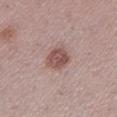The lesion was tiled from a total-body skin photograph and was not biopsied.
Imaged with white-light lighting.
The lesion's longest dimension is about 3.5 mm.
The total-body-photography lesion software estimated border irregularity of about 2 on a 0–10 scale, a within-lesion color-variation index near 3/10, and a peripheral color-asymmetry measure near 1. The analysis additionally found a classifier nevus-likeness of about 80/100 and a detector confidence of about 100 out of 100 that the crop contains a lesion.
The lesion is located on the left lower leg.
The patient is a female in their mid-50s.
A 15 mm close-up extracted from a 3D total-body photography capture.